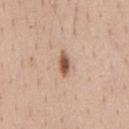Recorded during total-body skin imaging; not selected for excision or biopsy. Imaged with white-light lighting. A 15 mm close-up tile from a total-body photography series done for melanoma screening. A male patient, aged 38 to 42. On the chest. The recorded lesion diameter is about 3 mm.